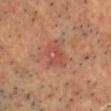This lesion was catalogued during total-body skin photography and was not selected for biopsy. The tile uses cross-polarized illumination. A subject roughly 55 years of age. The lesion's longest dimension is about 3.5 mm. Located on the front of the torso. A 15 mm close-up extracted from a 3D total-body photography capture.